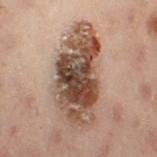Captured under cross-polarized illumination.
Approximately 10 mm at its widest.
Automated tile analysis of the lesion measured an eccentricity of roughly 0.9. It also reported a lesion color around L≈39 a*≈15 b*≈23 in CIELAB and a normalized border contrast of about 11.5.
Located on the left leg.
A 15 mm crop from a total-body photograph taken for skin-cancer surveillance.
A female subject, aged 53 to 57.